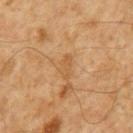follow-up: no biopsy performed (imaged during a skin exam)
body site: the mid back
patient: male, roughly 60 years of age
imaging modality: 15 mm crop, total-body photography
lesion diameter: about 3 mm